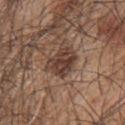Clinical impression: Captured during whole-body skin photography for melanoma surveillance; the lesion was not biopsied. Acquisition and patient details: The lesion is on the upper back. The patient is a male aged 43–47. A 15 mm crop from a total-body photograph taken for skin-cancer surveillance.